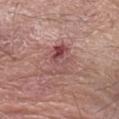Recorded during total-body skin imaging; not selected for excision or biopsy. From the left forearm. A male subject, aged 78 to 82. A close-up tile cropped from a whole-body skin photograph, about 15 mm across.